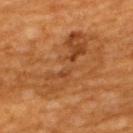About 12.5 mm across. This is a cross-polarized tile. A 15 mm crop from a total-body photograph taken for skin-cancer surveillance. A male subject, roughly 60 years of age. The lesion is located on the upper back.